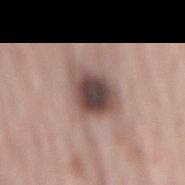workup — catalogued during a skin exam; not biopsied
imaging modality — ~15 mm crop, total-body skin-cancer survey
patient — male, in their mid- to late 60s
location — the mid back